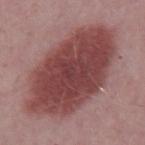Assessment: The lesion was photographed on a routine skin check and not biopsied; there is no pathology result. Background: On the mid back. The patient is a male about 50 years old. Cropped from a total-body skin-imaging series; the visible field is about 15 mm.